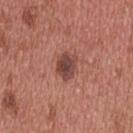{"biopsy_status": "not biopsied; imaged during a skin examination"}